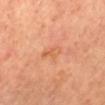{"biopsy_status": "not biopsied; imaged during a skin examination", "lesion_size": {"long_diameter_mm_approx": 3.0}, "lighting": "cross-polarized", "image": {"source": "total-body photography crop", "field_of_view_mm": 15}, "automated_metrics": {"nevus_likeness_0_100": 0}, "site": "mid back", "patient": {"sex": "female"}}